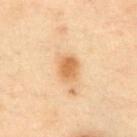Imaged during a routine full-body skin examination; the lesion was not biopsied and no histopathology is available. This is a cross-polarized tile. Cropped from a whole-body photographic skin survey; the tile spans about 15 mm. Located on the chest. The lesion-visualizer software estimated an automated nevus-likeness rating near 95 out of 100 and lesion-presence confidence of about 100/100. A male subject in their mid-50s.